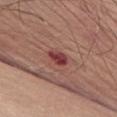Assessment:
The lesion was photographed on a routine skin check and not biopsied; there is no pathology result.
Image and clinical context:
Automated image analysis of the tile measured a classifier nevus-likeness of about 10/100 and a detector confidence of about 100 out of 100 that the crop contains a lesion. The lesion's longest dimension is about 3 mm. A region of skin cropped from a whole-body photographic capture, roughly 15 mm wide. Located on the abdomen. Imaged with white-light lighting. A male subject aged around 80.